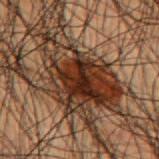notes: imaged on a skin check; not biopsied
subject: male, approximately 50 years of age
anatomic site: the mid back
acquisition: total-body-photography crop, ~15 mm field of view
diameter: ≈13.5 mm